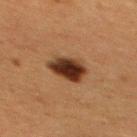Assessment: Part of a total-body skin-imaging series; this lesion was reviewed on a skin check and was not flagged for biopsy. Image and clinical context: This is a cross-polarized tile. A female subject, roughly 40 years of age. The lesion is on the upper back. An algorithmic analysis of the crop reported a footprint of about 10 mm², an eccentricity of roughly 0.6, and a symmetry-axis asymmetry near 0.25. And it measured a nevus-likeness score of about 95/100 and a lesion-detection confidence of about 100/100. A 15 mm close-up tile from a total-body photography series done for melanoma screening. The lesion's longest dimension is about 4 mm.